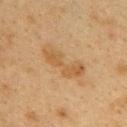Recorded during total-body skin imaging; not selected for excision or biopsy.
The lesion is on the upper back.
A lesion tile, about 15 mm wide, cut from a 3D total-body photograph.
This is a cross-polarized tile.
A female patient aged 38 to 42.
An algorithmic analysis of the crop reported an average lesion color of about L≈46 a*≈16 b*≈34 (CIELAB), roughly 7 lightness units darker than nearby skin, and a normalized lesion–skin contrast near 6.